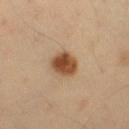Assessment: This lesion was catalogued during total-body skin photography and was not selected for biopsy. Acquisition and patient details: About 3.5 mm across. The total-body-photography lesion software estimated an automated nevus-likeness rating near 100 out of 100 and a detector confidence of about 100 out of 100 that the crop contains a lesion. The subject is a male about 40 years old. The lesion is on the leg. A 15 mm close-up tile from a total-body photography series done for melanoma screening.